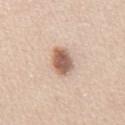Part of a total-body skin-imaging series; this lesion was reviewed on a skin check and was not flagged for biopsy.
A male subject, about 45 years old.
The lesion is located on the front of the torso.
The tile uses white-light illumination.
Automated tile analysis of the lesion measured a footprint of about 7.5 mm² and a shape-asymmetry score of about 0.15 (0 = symmetric). It also reported a lesion color around L≈60 a*≈19 b*≈28 in CIELAB and a normalized border contrast of about 10. The analysis additionally found border irregularity of about 1.5 on a 0–10 scale, a color-variation rating of about 5/10, and radial color variation of about 1.5. The software also gave an automated nevus-likeness rating near 100 out of 100.
About 4 mm across.
This image is a 15 mm lesion crop taken from a total-body photograph.